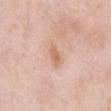Clinical impression:
Part of a total-body skin-imaging series; this lesion was reviewed on a skin check and was not flagged for biopsy.
Acquisition and patient details:
Located on the abdomen. The subject is a male aged 53 to 57. Longest diameter approximately 2.5 mm. Automated tile analysis of the lesion measured an area of roughly 3 mm², an eccentricity of roughly 0.85, and a symmetry-axis asymmetry near 0.3. The analysis additionally found a lesion color around L≈65 a*≈21 b*≈33 in CIELAB, about 9 CIELAB-L* units darker than the surrounding skin, and a normalized border contrast of about 6.5. The analysis additionally found a nevus-likeness score of about 0/100 and a lesion-detection confidence of about 100/100. Captured under white-light illumination. A lesion tile, about 15 mm wide, cut from a 3D total-body photograph.